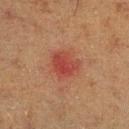Clinical impression: No biopsy was performed on this lesion — it was imaged during a full skin examination and was not determined to be concerning. Acquisition and patient details: The total-body-photography lesion software estimated an average lesion color of about L≈35 a*≈26 b*≈25 (CIELAB), about 7 CIELAB-L* units darker than the surrounding skin, and a normalized lesion–skin contrast near 7. And it measured radial color variation of about 1. On the right lower leg. A lesion tile, about 15 mm wide, cut from a 3D total-body photograph. The patient is a male in their 60s. Longest diameter approximately 3 mm.